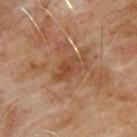biopsy_status: not biopsied; imaged during a skin examination
site: upper back
image:
  source: total-body photography crop
  field_of_view_mm: 15
lesion_size:
  long_diameter_mm_approx: 3.5
patient:
  sex: male
  age_approx: 65
automated_metrics:
  area_mm2_approx: 5.0
  eccentricity: 0.85
  shape_asymmetry: 0.25
  cielab_L: 44
  cielab_a: 22
  cielab_b: 32
  vs_skin_darker_L: 8.0
  vs_skin_contrast_norm: 6.0
  nevus_likeness_0_100: 0